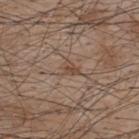Impression:
Imaged during a routine full-body skin examination; the lesion was not biopsied and no histopathology is available.
Background:
The lesion is located on the back. The patient is a male roughly 45 years of age. A 15 mm close-up extracted from a 3D total-body photography capture.